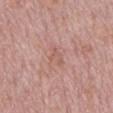Impression: This lesion was catalogued during total-body skin photography and was not selected for biopsy. Image and clinical context: An algorithmic analysis of the crop reported a nevus-likeness score of about 0/100 and a detector confidence of about 95 out of 100 that the crop contains a lesion. A region of skin cropped from a whole-body photographic capture, roughly 15 mm wide. A male patient about 75 years old. The lesion's longest dimension is about 3 mm. Imaged with white-light lighting. The lesion is on the back.